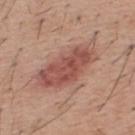Q: Was this lesion biopsied?
A: total-body-photography surveillance lesion; no biopsy
Q: What is the imaging modality?
A: ~15 mm crop, total-body skin-cancer survey
Q: Lesion size?
A: about 8 mm
Q: Illumination type?
A: white-light
Q: What are the patient's age and sex?
A: male, aged 38 to 42
Q: Where on the body is the lesion?
A: the upper back